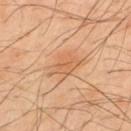This lesion was catalogued during total-body skin photography and was not selected for biopsy. Imaged with cross-polarized lighting. Located on the upper back. A close-up tile cropped from a whole-body skin photograph, about 15 mm across. Automated image analysis of the tile measured an area of roughly 10 mm², a shape eccentricity near 0.8, and two-axis asymmetry of about 0.3. The software also gave radial color variation of about 1. And it measured a classifier nevus-likeness of about 10/100 and a detector confidence of about 100 out of 100 that the crop contains a lesion. Approximately 4.5 mm at its widest. The subject is a male in their 50s.